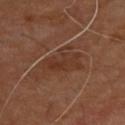Findings:
- biopsy status · imaged on a skin check; not biopsied
- imaging modality · 15 mm crop, total-body photography
- automated metrics · roughly 6 lightness units darker than nearby skin and a normalized border contrast of about 6; a lesion-detection confidence of about 90/100
- patient · male, in their 70s
- size · ≈5 mm
- tile lighting · cross-polarized
- site · the upper back